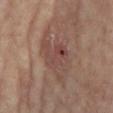biopsy status: imaged on a skin check; not biopsied
location: the right lower leg
size: ~7 mm (longest diameter)
acquisition: ~15 mm crop, total-body skin-cancer survey
patient: female, aged approximately 75